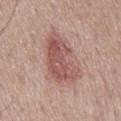workup: total-body-photography surveillance lesion; no biopsy
patient: male, aged 68–72
location: the back
image: total-body-photography crop, ~15 mm field of view